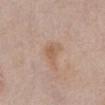No biopsy was performed on this lesion — it was imaged during a full skin examination and was not determined to be concerning. The lesion is on the front of the torso. A male subject, in their mid-70s. About 3.5 mm across. Cropped from a whole-body photographic skin survey; the tile spans about 15 mm. This is a white-light tile. Automated image analysis of the tile measured a mean CIELAB color near L≈58 a*≈18 b*≈30, a lesion–skin lightness drop of about 7, and a lesion-to-skin contrast of about 5.5 (normalized; higher = more distinct). It also reported a lesion-detection confidence of about 100/100.